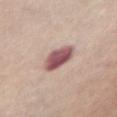No biopsy was performed on this lesion — it was imaged during a full skin examination and was not determined to be concerning. Located on the abdomen. A female patient about 55 years old. The lesion's longest dimension is about 4 mm. The tile uses white-light illumination. This image is a 15 mm lesion crop taken from a total-body photograph. The lesion-visualizer software estimated a lesion area of about 8 mm² and a symmetry-axis asymmetry near 0.2. And it measured an average lesion color of about L≈53 a*≈24 b*≈19 (CIELAB), a lesion–skin lightness drop of about 18, and a lesion-to-skin contrast of about 12.5 (normalized; higher = more distinct). The software also gave border irregularity of about 2 on a 0–10 scale, internal color variation of about 5.5 on a 0–10 scale, and peripheral color asymmetry of about 2. The analysis additionally found lesion-presence confidence of about 100/100.